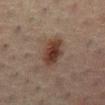Imaged with cross-polarized lighting.
On the abdomen.
The subject is a male roughly 50 years of age.
Longest diameter approximately 4.5 mm.
A 15 mm close-up tile from a total-body photography series done for melanoma screening.
An algorithmic analysis of the crop reported a lesion area of about 9.5 mm², an eccentricity of roughly 0.8, and a shape-asymmetry score of about 0.2 (0 = symmetric). The analysis additionally found a mean CIELAB color near L≈28 a*≈14 b*≈20 and roughly 9 lightness units darker than nearby skin. The analysis additionally found border irregularity of about 2 on a 0–10 scale, a within-lesion color-variation index near 4/10, and peripheral color asymmetry of about 1.